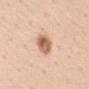Impression: Imaged during a routine full-body skin examination; the lesion was not biopsied and no histopathology is available. Context: On the right upper arm. Captured under white-light illumination. Automated image analysis of the tile measured an area of roughly 6.5 mm², a shape eccentricity near 0.9, and a symmetry-axis asymmetry near 0.15. The software also gave an average lesion color of about L≈63 a*≈21 b*≈33 (CIELAB), a lesion–skin lightness drop of about 16, and a lesion-to-skin contrast of about 9.5 (normalized; higher = more distinct). The analysis additionally found a border-irregularity rating of about 2/10 and radial color variation of about 1.5. A 15 mm crop from a total-body photograph taken for skin-cancer surveillance. A male subject roughly 50 years of age.